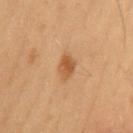<case>
<biopsy_status>not biopsied; imaged during a skin examination</biopsy_status>
</case>